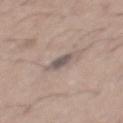Q: Was this lesion biopsied?
A: total-body-photography surveillance lesion; no biopsy
Q: Lesion size?
A: ~2.5 mm (longest diameter)
Q: Illumination type?
A: white-light
Q: What kind of image is this?
A: 15 mm crop, total-body photography
Q: Automated lesion metrics?
A: a lesion area of about 3.5 mm², a shape eccentricity near 0.8, and a symmetry-axis asymmetry near 0.15; a normalized border contrast of about 9; a nevus-likeness score of about 0/100 and lesion-presence confidence of about 75/100
Q: Patient demographics?
A: male, aged approximately 65
Q: What is the anatomic site?
A: the back